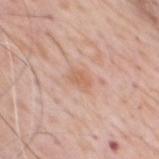| feature | finding |
|---|---|
| subject | male, roughly 80 years of age |
| site | the chest |
| image | ~15 mm tile from a whole-body skin photo |